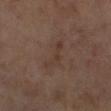| feature | finding |
|---|---|
| follow-up | no biopsy performed (imaged during a skin exam) |
| patient | female, in their 60s |
| diameter | ≈4 mm |
| automated lesion analysis | a shape eccentricity near 0.95 and a shape-asymmetry score of about 0.6 (0 = symmetric); a lesion–skin lightness drop of about 5; a within-lesion color-variation index near 0/10; a nevus-likeness score of about 0/100 and a lesion-detection confidence of about 100/100 |
| site | the right lower leg |
| imaging modality | ~15 mm crop, total-body skin-cancer survey |
| tile lighting | cross-polarized |